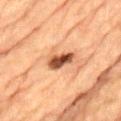Longest diameter approximately 3 mm. Captured under cross-polarized illumination. A male patient aged around 85 or older. The lesion-visualizer software estimated a lesion area of about 5.5 mm² and a shape eccentricity near 0.8. And it measured a border-irregularity index near 2/10. A 15 mm close-up tile from a total-body photography series done for melanoma screening. Located on the lower back.